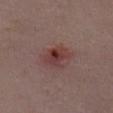Cropped from a total-body skin-imaging series; the visible field is about 15 mm. Longest diameter approximately 3.5 mm. A female subject approximately 35 years of age. The lesion is on the chest. Captured under white-light illumination.